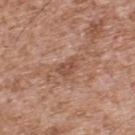Impression:
Captured during whole-body skin photography for melanoma surveillance; the lesion was not biopsied.
Clinical summary:
Approximately 2.5 mm at its widest. A roughly 15 mm field-of-view crop from a total-body skin photograph. The lesion-visualizer software estimated a nevus-likeness score of about 0/100 and lesion-presence confidence of about 100/100. Located on the upper back. A male subject, roughly 55 years of age.